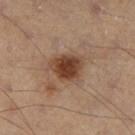Q: Is there a histopathology result?
A: no biopsy performed (imaged during a skin exam)
Q: Where on the body is the lesion?
A: the left leg
Q: What is the imaging modality?
A: 15 mm crop, total-body photography
Q: What are the patient's age and sex?
A: male, approximately 50 years of age
Q: Automated lesion metrics?
A: a footprint of about 9.5 mm² and a shape-asymmetry score of about 0.25 (0 = symmetric); an automated nevus-likeness rating near 95 out of 100
Q: What is the lesion's diameter?
A: ~4 mm (longest diameter)
Q: How was the tile lit?
A: cross-polarized illumination